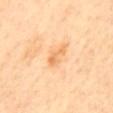Recorded during total-body skin imaging; not selected for excision or biopsy. A region of skin cropped from a whole-body photographic capture, roughly 15 mm wide. On the mid back. The patient is a female approximately 70 years of age. Measured at roughly 3.5 mm in maximum diameter.